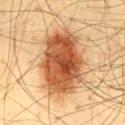* image · total-body-photography crop, ~15 mm field of view
* lesion size · ~8.5 mm (longest diameter)
* subject · male, about 45 years old
* site · the mid back